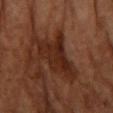| feature | finding |
|---|---|
| biopsy status | imaged on a skin check; not biopsied |
| lighting | cross-polarized |
| location | the right forearm |
| subject | female, aged 63–67 |
| diameter | about 6 mm |
| acquisition | ~15 mm tile from a whole-body skin photo |